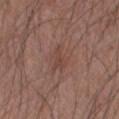No biopsy was performed on this lesion — it was imaged during a full skin examination and was not determined to be concerning.
From the left forearm.
This image is a 15 mm lesion crop taken from a total-body photograph.
The patient is a male aged 43–47.
Captured under white-light illumination.